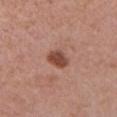Q: Is there a histopathology result?
A: no biopsy performed (imaged during a skin exam)
Q: Illumination type?
A: white-light
Q: What is the imaging modality?
A: 15 mm crop, total-body photography
Q: Lesion location?
A: the chest
Q: What are the patient's age and sex?
A: female, about 60 years old